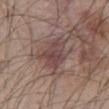Impression: The lesion was photographed on a routine skin check and not biopsied; there is no pathology result. Context: A 15 mm close-up extracted from a 3D total-body photography capture. The subject is a male in their mid-40s. From the abdomen.